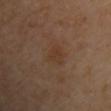This lesion was catalogued during total-body skin photography and was not selected for biopsy. The lesion is on the arm. Imaged with cross-polarized lighting. A region of skin cropped from a whole-body photographic capture, roughly 15 mm wide. The subject is a female roughly 65 years of age. Automated tile analysis of the lesion measured a border-irregularity index near 3/10, internal color variation of about 2.5 on a 0–10 scale, and peripheral color asymmetry of about 1. And it measured a nevus-likeness score of about 5/100 and a lesion-detection confidence of about 100/100. The lesion's longest dimension is about 3 mm.